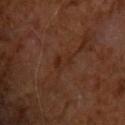Impression:
Captured during whole-body skin photography for melanoma surveillance; the lesion was not biopsied.
Acquisition and patient details:
On the upper back. A male subject, aged around 65. Captured under cross-polarized illumination. A 15 mm crop from a total-body photograph taken for skin-cancer surveillance. Automated tile analysis of the lesion measured an area of roughly 3.5 mm², an outline eccentricity of about 0.85 (0 = round, 1 = elongated), and a shape-asymmetry score of about 0.5 (0 = symmetric). It also reported lesion-presence confidence of about 100/100.